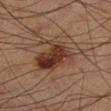Recorded during total-body skin imaging; not selected for excision or biopsy. This image is a 15 mm lesion crop taken from a total-body photograph. Measured at roughly 7 mm in maximum diameter. This is a cross-polarized tile. The patient is a male aged around 60. The lesion-visualizer software estimated an outline eccentricity of about 0.8 (0 = round, 1 = elongated) and a symmetry-axis asymmetry near 0.35. The software also gave a lesion color around L≈36 a*≈19 b*≈26 in CIELAB, roughly 11 lightness units darker than nearby skin, and a normalized border contrast of about 9.5. It also reported an automated nevus-likeness rating near 65 out of 100. From the left lower leg.